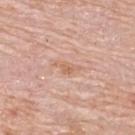Part of a total-body skin-imaging series; this lesion was reviewed on a skin check and was not flagged for biopsy.
The lesion-visualizer software estimated an average lesion color of about L≈64 a*≈21 b*≈32 (CIELAB) and a normalized border contrast of about 6. The analysis additionally found an automated nevus-likeness rating near 0 out of 100.
Cropped from a total-body skin-imaging series; the visible field is about 15 mm.
Imaged with white-light lighting.
A female subject, aged approximately 65.
The lesion is located on the back.